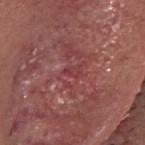| feature | finding |
|---|---|
| biopsy status | total-body-photography surveillance lesion; no biopsy |
| size | ~2 mm (longest diameter) |
| location | the head or neck |
| tile lighting | white-light |
| acquisition | 15 mm crop, total-body photography |
| patient | male, aged approximately 70 |